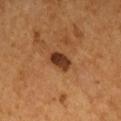Clinical summary: An algorithmic analysis of the crop reported border irregularity of about 1.5 on a 0–10 scale, a color-variation rating of about 4.5/10, and peripheral color asymmetry of about 1.5. The software also gave a classifier nevus-likeness of about 60/100 and a detector confidence of about 100 out of 100 that the crop contains a lesion. A region of skin cropped from a whole-body photographic capture, roughly 15 mm wide. Located on the right upper arm. Imaged with cross-polarized lighting. A male patient aged 53 to 57. Longest diameter approximately 3 mm.